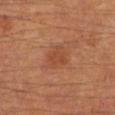{"image": {"source": "total-body photography crop", "field_of_view_mm": 15}, "patient": {"sex": "male", "age_approx": 65}, "site": "left lower leg"}